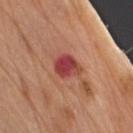Impression: Captured during whole-body skin photography for melanoma surveillance; the lesion was not biopsied. Acquisition and patient details: A male patient, roughly 70 years of age. Longest diameter approximately 3 mm. The tile uses white-light illumination. Cropped from a whole-body photographic skin survey; the tile spans about 15 mm. An algorithmic analysis of the crop reported two-axis asymmetry of about 0.2. The analysis additionally found border irregularity of about 2 on a 0–10 scale, internal color variation of about 7 on a 0–10 scale, and peripheral color asymmetry of about 2.5. It also reported lesion-presence confidence of about 100/100. The lesion is on the left upper arm.